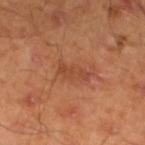Recorded during total-body skin imaging; not selected for excision or biopsy.